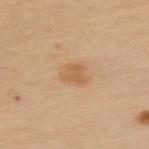Q: Was a biopsy performed?
A: no biopsy performed (imaged during a skin exam)
Q: What is the lesion's diameter?
A: ~3 mm (longest diameter)
Q: Lesion location?
A: the left upper arm
Q: What is the imaging modality?
A: 15 mm crop, total-body photography
Q: What did automated image analysis measure?
A: a lesion area of about 4.5 mm² and a shape-asymmetry score of about 0.35 (0 = symmetric); a detector confidence of about 100 out of 100 that the crop contains a lesion
Q: How was the tile lit?
A: cross-polarized illumination
Q: Patient demographics?
A: female, aged 48 to 52